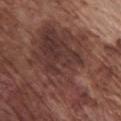Clinical impression:
No biopsy was performed on this lesion — it was imaged during a full skin examination and was not determined to be concerning.
Background:
A male subject roughly 75 years of age. Imaged with white-light lighting. The lesion is on the chest. Measured at roughly 12 mm in maximum diameter. This image is a 15 mm lesion crop taken from a total-body photograph.